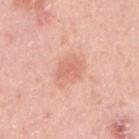Imaged during a routine full-body skin examination; the lesion was not biopsied and no histopathology is available.
Imaged with white-light lighting.
A region of skin cropped from a whole-body photographic capture, roughly 15 mm wide.
A female subject, aged 28 to 32.
Approximately 3 mm at its widest.
From the right upper arm.